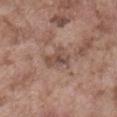Imaged during a routine full-body skin examination; the lesion was not biopsied and no histopathology is available. Approximately 3.5 mm at its widest. A male subject, roughly 75 years of age. This image is a 15 mm lesion crop taken from a total-body photograph. The lesion is located on the abdomen.